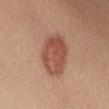follow-up: total-body-photography surveillance lesion; no biopsy | anatomic site: the front of the torso | subject: female, in their mid- to late 40s | lighting: white-light | image source: ~15 mm tile from a whole-body skin photo.